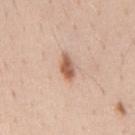Impression:
Recorded during total-body skin imaging; not selected for excision or biopsy.
Background:
A lesion tile, about 15 mm wide, cut from a 3D total-body photograph. Located on the mid back. A male subject aged 38–42. Imaged with white-light lighting. Automated tile analysis of the lesion measured a lesion color around L≈60 a*≈22 b*≈31 in CIELAB and a lesion-to-skin contrast of about 9 (normalized; higher = more distinct). And it measured border irregularity of about 2.5 on a 0–10 scale, internal color variation of about 4 on a 0–10 scale, and peripheral color asymmetry of about 1.5.